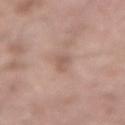notes: imaged on a skin check; not biopsied | TBP lesion metrics: a mean CIELAB color near L≈56 a*≈18 b*≈25 and a lesion–skin lightness drop of about 9; a border-irregularity rating of about 4/10, a within-lesion color-variation index near 0.5/10, and radial color variation of about 0; a nevus-likeness score of about 0/100 and a detector confidence of about 90 out of 100 that the crop contains a lesion | lesion diameter: about 2.5 mm | site: the lower back | subject: male, aged approximately 55 | image source: ~15 mm tile from a whole-body skin photo.